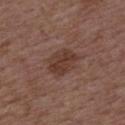{"biopsy_status": "not biopsied; imaged during a skin examination"}